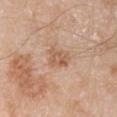notes: imaged on a skin check; not biopsied | lighting: white-light illumination | image source: ~15 mm tile from a whole-body skin photo | subject: male, aged around 80 | diameter: ~3 mm (longest diameter) | image-analysis metrics: a lesion color around L≈58 a*≈20 b*≈32 in CIELAB and roughly 9 lightness units darker than nearby skin; border irregularity of about 3 on a 0–10 scale, internal color variation of about 3.5 on a 0–10 scale, and radial color variation of about 1.5; lesion-presence confidence of about 100/100 | site: the right upper arm.